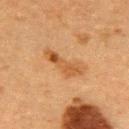notes = no biopsy performed (imaged during a skin exam); subject = female, roughly 55 years of age; lighting = cross-polarized; site = the upper back; acquisition = 15 mm crop, total-body photography.